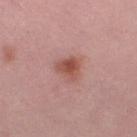- workup — catalogued during a skin exam; not biopsied
- location — the right lower leg
- diameter — ≈3 mm
- acquisition — ~15 mm tile from a whole-body skin photo
- subject — female, approximately 40 years of age